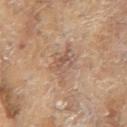The lesion was photographed on a routine skin check and not biopsied; there is no pathology result. A 15 mm close-up extracted from a 3D total-body photography capture. An algorithmic analysis of the crop reported border irregularity of about 5 on a 0–10 scale, internal color variation of about 4.5 on a 0–10 scale, and a peripheral color-asymmetry measure near 1.5. The analysis additionally found a nevus-likeness score of about 0/100 and a detector confidence of about 95 out of 100 that the crop contains a lesion. Imaged with cross-polarized lighting. The subject is a female roughly 80 years of age. On the right arm.